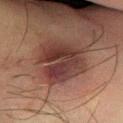Recorded during total-body skin imaging; not selected for excision or biopsy. Approximately 5.5 mm at its widest. Cropped from a total-body skin-imaging series; the visible field is about 15 mm. A male subject, aged 63–67. On the left lower leg.